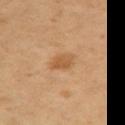Clinical impression:
Imaged during a routine full-body skin examination; the lesion was not biopsied and no histopathology is available.
Clinical summary:
The tile uses cross-polarized illumination. A male patient aged approximately 55. The lesion's longest dimension is about 3 mm. Automated tile analysis of the lesion measured a border-irregularity rating of about 2/10, a within-lesion color-variation index near 2/10, and peripheral color asymmetry of about 1. This image is a 15 mm lesion crop taken from a total-body photograph. The lesion is on the right upper arm.